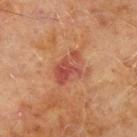biopsy_status: not biopsied; imaged during a skin examination
lighting: cross-polarized
site: left upper arm
patient:
  sex: male
  age_approx: 70
image:
  source: total-body photography crop
  field_of_view_mm: 15
automated_metrics:
  cielab_L: 41
  cielab_a: 24
  cielab_b: 26
  vs_skin_darker_L: 8.0
  vs_skin_contrast_norm: 7.0
  border_irregularity_0_10: 2.5
  peripheral_color_asymmetry: 2.0
  nevus_likeness_0_100: 0
  lesion_detection_confidence_0_100: 100
lesion_size:
  long_diameter_mm_approx: 4.0